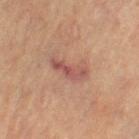This lesion was catalogued during total-body skin photography and was not selected for biopsy. A female subject aged 63 to 67. From the left thigh. The recorded lesion diameter is about 4.5 mm. A close-up tile cropped from a whole-body skin photograph, about 15 mm across. This is a cross-polarized tile.